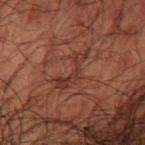biopsy_status: not biopsied; imaged during a skin examination
patient:
  sex: male
  age_approx: 50
image:
  source: total-body photography crop
  field_of_view_mm: 15
site: right lower leg